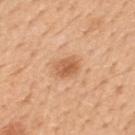Clinical impression: Captured during whole-body skin photography for melanoma surveillance; the lesion was not biopsied. Acquisition and patient details: An algorithmic analysis of the crop reported a footprint of about 5 mm², a shape eccentricity near 0.65, and a shape-asymmetry score of about 0.15 (0 = symmetric). The analysis additionally found a border-irregularity index near 1.5/10, internal color variation of about 4 on a 0–10 scale, and peripheral color asymmetry of about 1.5. And it measured a nevus-likeness score of about 85/100 and a lesion-detection confidence of about 100/100. Measured at roughly 2.5 mm in maximum diameter. A male subject aged approximately 50. From the mid back. A 15 mm close-up extracted from a 3D total-body photography capture. Captured under white-light illumination.